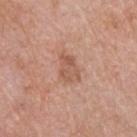| feature | finding |
|---|---|
| notes | total-body-photography surveillance lesion; no biopsy |
| subject | male, in their 50s |
| acquisition | ~15 mm tile from a whole-body skin photo |
| illumination | white-light |
| site | the chest |